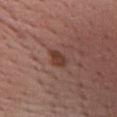Clinical impression:
Imaged during a routine full-body skin examination; the lesion was not biopsied and no histopathology is available.
Image and clinical context:
A female patient, in their mid-50s. On the chest. A 15 mm close-up extracted from a 3D total-body photography capture.